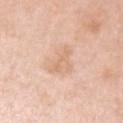Q: Where on the body is the lesion?
A: the left upper arm
Q: Illumination type?
A: white-light illumination
Q: Who is the patient?
A: female, aged approximately 40
Q: Automated lesion metrics?
A: an area of roughly 8.5 mm² and a symmetry-axis asymmetry near 0.4; a mean CIELAB color near L≈71 a*≈19 b*≈32, a lesion–skin lightness drop of about 7, and a lesion-to-skin contrast of about 5 (normalized; higher = more distinct); a border-irregularity index near 4.5/10 and a color-variation rating of about 2.5/10; an automated nevus-likeness rating near 0 out of 100 and a lesion-detection confidence of about 100/100
Q: How was this image acquired?
A: 15 mm crop, total-body photography